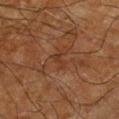{
  "site": "right lower leg",
  "patient": {
    "sex": "male",
    "age_approx": 65
  },
  "image": {
    "source": "total-body photography crop",
    "field_of_view_mm": 15
  }
}